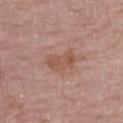Q: Was this lesion biopsied?
A: imaged on a skin check; not biopsied
Q: What kind of image is this?
A: ~15 mm crop, total-body skin-cancer survey
Q: What are the patient's age and sex?
A: female, about 65 years old
Q: Lesion size?
A: about 4.5 mm
Q: Illumination type?
A: white-light illumination
Q: Lesion location?
A: the left thigh